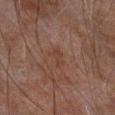Impression: This lesion was catalogued during total-body skin photography and was not selected for biopsy. Acquisition and patient details: On the arm. Measured at roughly 2.5 mm in maximum diameter. Imaged with cross-polarized lighting. A male subject about 60 years old. A roughly 15 mm field-of-view crop from a total-body skin photograph. The lesion-visualizer software estimated an area of roughly 2.5 mm², an outline eccentricity of about 0.9 (0 = round, 1 = elongated), and a shape-asymmetry score of about 0.45 (0 = symmetric). The software also gave border irregularity of about 5 on a 0–10 scale, a within-lesion color-variation index near 0/10, and a peripheral color-asymmetry measure near 0.